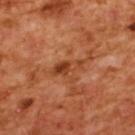Impression:
No biopsy was performed on this lesion — it was imaged during a full skin examination and was not determined to be concerning.
Clinical summary:
Automated image analysis of the tile measured a footprint of about 5 mm², a shape eccentricity near 0.9, and two-axis asymmetry of about 0.35. It also reported a classifier nevus-likeness of about 0/100. A female patient, aged 53 to 57. The lesion's longest dimension is about 4 mm. This image is a 15 mm lesion crop taken from a total-body photograph. The lesion is located on the upper back.